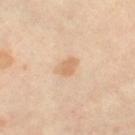Recorded during total-body skin imaging; not selected for excision or biopsy. On the right thigh. The tile uses cross-polarized illumination. Cropped from a whole-body photographic skin survey; the tile spans about 15 mm. A female subject about 50 years old. About 2.5 mm across.